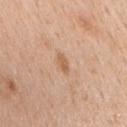Image and clinical context: The lesion's longest dimension is about 2.5 mm. The lesion-visualizer software estimated a shape eccentricity near 0.9 and two-axis asymmetry of about 0.35. It also reported internal color variation of about 1 on a 0–10 scale. The software also gave an automated nevus-likeness rating near 0 out of 100. A male subject aged approximately 65. A 15 mm close-up tile from a total-body photography series done for melanoma screening. The lesion is located on the mid back. This is a white-light tile.